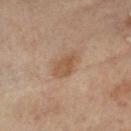Clinical summary: From the right lower leg. The patient is a female in their mid-70s. This image is a 15 mm lesion crop taken from a total-body photograph. Automated image analysis of the tile measured a within-lesion color-variation index near 2.5/10. The tile uses cross-polarized illumination.